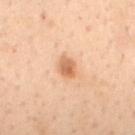Case summary:
- workup · no biopsy performed (imaged during a skin exam)
- body site · the mid back
- subject · female, in their mid- to late 50s
- acquisition · ~15 mm crop, total-body skin-cancer survey
- image-analysis metrics · a footprint of about 4.5 mm², a shape eccentricity near 0.55, and a shape-asymmetry score of about 0.15 (0 = symmetric); a lesion-detection confidence of about 100/100
- diameter · ≈2.5 mm